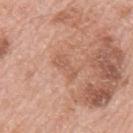Assessment: This lesion was catalogued during total-body skin photography and was not selected for biopsy. Clinical summary: The patient is a male in their 80s. The lesion-visualizer software estimated an area of roughly 4.5 mm² and a shape eccentricity near 0.9. And it measured an average lesion color of about L≈58 a*≈23 b*≈31 (CIELAB), roughly 7 lightness units darker than nearby skin, and a normalized border contrast of about 5. The analysis additionally found border irregularity of about 4 on a 0–10 scale, internal color variation of about 1.5 on a 0–10 scale, and a peripheral color-asymmetry measure near 0.5. The software also gave a classifier nevus-likeness of about 0/100 and a lesion-detection confidence of about 100/100. The lesion is on the left upper arm. Longest diameter approximately 3 mm. The tile uses white-light illumination. A region of skin cropped from a whole-body photographic capture, roughly 15 mm wide.